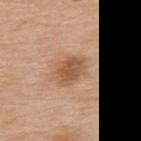A 15 mm close-up extracted from a 3D total-body photography capture. A female patient aged around 75. From the upper back.